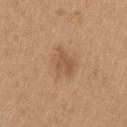Case summary:
- follow-up — catalogued during a skin exam; not biopsied
- imaging modality — total-body-photography crop, ~15 mm field of view
- anatomic site — the left upper arm
- subject — female, approximately 45 years of age